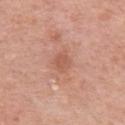workup — total-body-photography surveillance lesion; no biopsy
patient — female, in their mid-60s
diameter — ~2.5 mm (longest diameter)
anatomic site — the left upper arm
image source — 15 mm crop, total-body photography
TBP lesion metrics — border irregularity of about 2.5 on a 0–10 scale, a within-lesion color-variation index near 1.5/10, and radial color variation of about 0.5